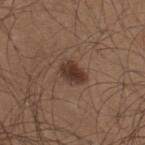Case summary:
- follow-up — total-body-photography surveillance lesion; no biopsy
- diameter — ~3.5 mm (longest diameter)
- imaging modality — ~15 mm crop, total-body skin-cancer survey
- body site — the upper back
- subject — male, aged approximately 25
- TBP lesion metrics — a footprint of about 6 mm², a shape eccentricity near 0.75, and a shape-asymmetry score of about 0.25 (0 = symmetric); about 11 CIELAB-L* units darker than the surrounding skin and a normalized lesion–skin contrast near 10; a border-irregularity index near 2/10; an automated nevus-likeness rating near 95 out of 100 and lesion-presence confidence of about 100/100
- tile lighting — white-light